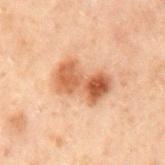Case summary:
• notes — no biopsy performed (imaged during a skin exam)
• lesion diameter — ≈5.5 mm
• site — the mid back
• acquisition — total-body-photography crop, ~15 mm field of view
• patient — male, roughly 70 years of age
• illumination — cross-polarized
• automated metrics — a mean CIELAB color near L≈46 a*≈20 b*≈30, a lesion–skin lightness drop of about 11, and a lesion-to-skin contrast of about 9 (normalized; higher = more distinct); a within-lesion color-variation index near 7.5/10; lesion-presence confidence of about 100/100